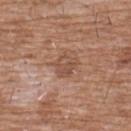Clinical impression: Recorded during total-body skin imaging; not selected for excision or biopsy. Clinical summary: A close-up tile cropped from a whole-body skin photograph, about 15 mm across. Measured at roughly 3 mm in maximum diameter. The lesion is on the chest. A male subject approximately 60 years of age.